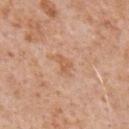Impression: No biopsy was performed on this lesion — it was imaged during a full skin examination and was not determined to be concerning. Context: Cropped from a whole-body photographic skin survey; the tile spans about 15 mm. From the front of the torso. The subject is a male in their mid-60s. This is a white-light tile. The total-body-photography lesion software estimated a lesion area of about 3 mm² and a symmetry-axis asymmetry near 0.35. The software also gave a mean CIELAB color near L≈60 a*≈22 b*≈35 and a lesion-to-skin contrast of about 5.5 (normalized; higher = more distinct).